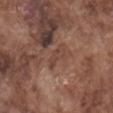The lesion was photographed on a routine skin check and not biopsied; there is no pathology result. A 15 mm close-up tile from a total-body photography series done for melanoma screening. The patient is a male aged 73–77. The recorded lesion diameter is about 3 mm. The lesion is on the mid back.